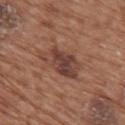This lesion was catalogued during total-body skin photography and was not selected for biopsy. The lesion is located on the back. A female subject, in their mid- to late 70s. This is a white-light tile. A roughly 15 mm field-of-view crop from a total-body skin photograph. Measured at roughly 5.5 mm in maximum diameter.